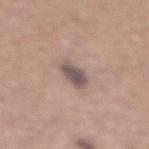follow-up: imaged on a skin check; not biopsied
size: ≈3.5 mm
site: the mid back
illumination: white-light
image source: 15 mm crop, total-body photography
subject: male, aged 43 to 47
automated lesion analysis: an area of roughly 5.5 mm², an eccentricity of roughly 0.8, and two-axis asymmetry of about 0.25; a lesion color around L≈52 a*≈13 b*≈18 in CIELAB, about 13 CIELAB-L* units darker than the surrounding skin, and a lesion-to-skin contrast of about 10 (normalized; higher = more distinct); a border-irregularity rating of about 2.5/10, internal color variation of about 3 on a 0–10 scale, and peripheral color asymmetry of about 1